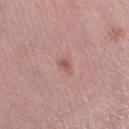notes = catalogued during a skin exam; not biopsied | anatomic site = the right lower leg | subject = female, approximately 50 years of age | size = ≈2.5 mm | automated lesion analysis = an area of roughly 3 mm² and an eccentricity of roughly 0.8; border irregularity of about 2.5 on a 0–10 scale and peripheral color asymmetry of about 0.5; an automated nevus-likeness rating near 15 out of 100 | lighting = white-light | image source = 15 mm crop, total-body photography.